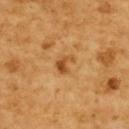Q: Was a biopsy performed?
A: imaged on a skin check; not biopsied
Q: Where on the body is the lesion?
A: the upper back
Q: Patient demographics?
A: female, roughly 55 years of age
Q: Lesion size?
A: about 2.5 mm
Q: Automated lesion metrics?
A: roughly 11 lightness units darker than nearby skin and a normalized lesion–skin contrast near 7.5; a classifier nevus-likeness of about 40/100 and a detector confidence of about 100 out of 100 that the crop contains a lesion
Q: What lighting was used for the tile?
A: cross-polarized illumination
Q: What kind of image is this?
A: total-body-photography crop, ~15 mm field of view